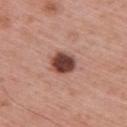The lesion is on the upper back. The subject is a male aged 58–62. A 15 mm close-up extracted from a 3D total-body photography capture.